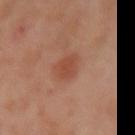Captured during whole-body skin photography for melanoma surveillance; the lesion was not biopsied.
A female patient aged approximately 40.
Cropped from a whole-body photographic skin survey; the tile spans about 15 mm.
On the left arm.
About 2.5 mm across.
Automated tile analysis of the lesion measured an area of roughly 5 mm², an eccentricity of roughly 0.4, and two-axis asymmetry of about 0.25. And it measured a mean CIELAB color near L≈48 a*≈26 b*≈32, about 8 CIELAB-L* units darker than the surrounding skin, and a lesion-to-skin contrast of about 6 (normalized; higher = more distinct). And it measured an automated nevus-likeness rating near 55 out of 100 and a detector confidence of about 100 out of 100 that the crop contains a lesion.
This is a cross-polarized tile.